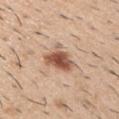Part of a total-body skin-imaging series; this lesion was reviewed on a skin check and was not flagged for biopsy. The lesion is located on the left upper arm. This is a white-light tile. A male subject aged 58 to 62. A 15 mm crop from a total-body photograph taken for skin-cancer surveillance. The lesion-visualizer software estimated radial color variation of about 1.5.